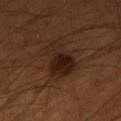biopsy status = no biopsy performed (imaged during a skin exam); automated lesion analysis = a mean CIELAB color near L≈21 a*≈16 b*≈21, about 8 CIELAB-L* units darker than the surrounding skin, and a normalized border contrast of about 9.5; site = the right thigh; lesion size = ~5 mm (longest diameter); acquisition = 15 mm crop, total-body photography; subject = male, in their mid- to late 50s.